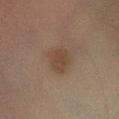Clinical impression: The lesion was photographed on a routine skin check and not biopsied; there is no pathology result. Context: Imaged with cross-polarized lighting. Cropped from a whole-body photographic skin survey; the tile spans about 15 mm. The lesion is located on the right lower leg. The subject is a male in their mid-40s.